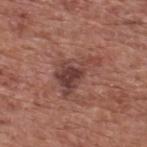Part of a total-body skin-imaging series; this lesion was reviewed on a skin check and was not flagged for biopsy. Located on the upper back. The tile uses white-light illumination. The subject is a male aged around 75. Longest diameter approximately 5.5 mm. Cropped from a whole-body photographic skin survey; the tile spans about 15 mm. The lesion-visualizer software estimated an area of roughly 11 mm², an eccentricity of roughly 0.8, and a symmetry-axis asymmetry near 0.55. The analysis additionally found border irregularity of about 7 on a 0–10 scale and peripheral color asymmetry of about 1.5.